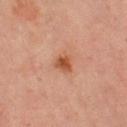This lesion was catalogued during total-body skin photography and was not selected for biopsy.
The subject is a female aged approximately 70.
Approximately 2.5 mm at its widest.
The lesion-visualizer software estimated a lesion color around L≈46 a*≈23 b*≈31 in CIELAB and about 9 CIELAB-L* units darker than the surrounding skin.
A 15 mm crop from a total-body photograph taken for skin-cancer surveillance.
Located on the leg.
Imaged with cross-polarized lighting.